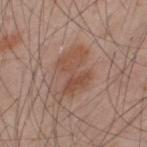Captured during whole-body skin photography for melanoma surveillance; the lesion was not biopsied. The lesion is on the upper back. The subject is a male aged 33 to 37. A close-up tile cropped from a whole-body skin photograph, about 15 mm across.